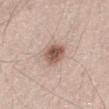lesion diameter: ~3.5 mm (longest diameter) | image source: ~15 mm tile from a whole-body skin photo | location: the abdomen | tile lighting: white-light illumination | image-analysis metrics: a footprint of about 7 mm², a shape eccentricity near 0.5, and a shape-asymmetry score of about 0.15 (0 = symmetric); a color-variation rating of about 4/10 and peripheral color asymmetry of about 1.5 | patient: male, in their mid- to late 60s.